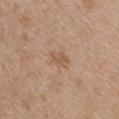No biopsy was performed on this lesion — it was imaged during a full skin examination and was not determined to be concerning. A female subject, aged 43 to 47. On the upper back. Cropped from a total-body skin-imaging series; the visible field is about 15 mm.